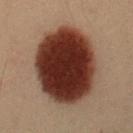Clinical impression: Recorded during total-body skin imaging; not selected for excision or biopsy. Context: This is a cross-polarized tile. Approximately 8 mm at its widest. Automated tile analysis of the lesion measured a mean CIELAB color near L≈25 a*≈19 b*≈22, a lesion–skin lightness drop of about 20, and a normalized border contrast of about 18. The analysis additionally found border irregularity of about 1 on a 0–10 scale, internal color variation of about 4.5 on a 0–10 scale, and radial color variation of about 1. The software also gave an automated nevus-likeness rating near 100 out of 100. A close-up tile cropped from a whole-body skin photograph, about 15 mm across. A male patient, aged 53–57. The lesion is on the arm.